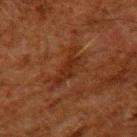Q: Is there a histopathology result?
A: total-body-photography surveillance lesion; no biopsy
Q: Lesion size?
A: ≈6 mm
Q: How was this image acquired?
A: total-body-photography crop, ~15 mm field of view
Q: Patient demographics?
A: male, approximately 60 years of age
Q: Illumination type?
A: cross-polarized
Q: Where on the body is the lesion?
A: the upper back